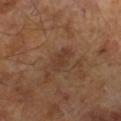Notes:
– notes — catalogued during a skin exam; not biopsied
– lighting — cross-polarized
– image — total-body-photography crop, ~15 mm field of view
– subject — male, aged around 65
– TBP lesion metrics — an area of roughly 5 mm², an eccentricity of roughly 0.75, and a symmetry-axis asymmetry near 0.2; a border-irregularity index near 2.5/10, internal color variation of about 1.5 on a 0–10 scale, and peripheral color asymmetry of about 0.5; an automated nevus-likeness rating near 0 out of 100